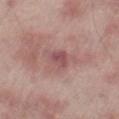Findings:
- imaging modality: total-body-photography crop, ~15 mm field of view
- patient: male, in their mid-60s
- body site: the leg
- tile lighting: white-light illumination